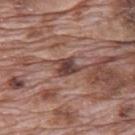This lesion was catalogued during total-body skin photography and was not selected for biopsy.
This is a white-light tile.
A male subject aged approximately 70.
The lesion-visualizer software estimated a footprint of about 4 mm², an outline eccentricity of about 0.5 (0 = round, 1 = elongated), and two-axis asymmetry of about 0.35. It also reported a border-irregularity index near 3/10 and a peripheral color-asymmetry measure near 1. It also reported an automated nevus-likeness rating near 0 out of 100 and lesion-presence confidence of about 95/100.
Located on the mid back.
A close-up tile cropped from a whole-body skin photograph, about 15 mm across.
The lesion's longest dimension is about 2.5 mm.